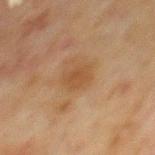biopsy status: catalogued during a skin exam; not biopsied
patient: male, aged approximately 70
diameter: ~2.5 mm (longest diameter)
lighting: cross-polarized
image: total-body-photography crop, ~15 mm field of view
anatomic site: the mid back